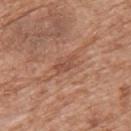Captured during whole-body skin photography for melanoma surveillance; the lesion was not biopsied. A close-up tile cropped from a whole-body skin photograph, about 15 mm across. The lesion is on the upper back. A male patient, roughly 60 years of age.